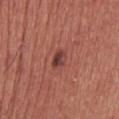- workup: total-body-photography surveillance lesion; no biopsy
- acquisition: total-body-photography crop, ~15 mm field of view
- lighting: white-light illumination
- patient: male, aged around 60
- diameter: ~2.5 mm (longest diameter)
- body site: the head or neck